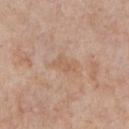The lesion was photographed on a routine skin check and not biopsied; there is no pathology result. Imaged with white-light lighting. The subject is a male in their mid- to late 50s. The lesion is on the chest. Measured at roughly 3 mm in maximum diameter. The total-body-photography lesion software estimated an average lesion color of about L≈59 a*≈19 b*≈31 (CIELAB), roughly 6 lightness units darker than nearby skin, and a normalized border contrast of about 5. And it measured a border-irregularity index near 5/10 and internal color variation of about 1.5 on a 0–10 scale. This image is a 15 mm lesion crop taken from a total-body photograph.